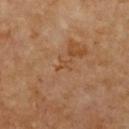follow-up = imaged on a skin check; not biopsied
location = the chest
subject = female, in their mid- to late 60s
lesion diameter = about 2.5 mm
tile lighting = cross-polarized illumination
image source = ~15 mm tile from a whole-body skin photo